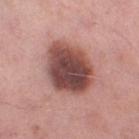{"biopsy_status": "not biopsied; imaged during a skin examination", "lesion_size": {"long_diameter_mm_approx": 6.5}, "lighting": "white-light", "image": {"source": "total-body photography crop", "field_of_view_mm": 15}, "patient": {"sex": "male", "age_approx": 55}, "site": "right lower leg", "automated_metrics": {"area_mm2_approx": 25.0, "eccentricity": 0.65, "cielab_L": 47, "cielab_a": 23, "cielab_b": 22, "vs_skin_contrast_norm": 12.0, "border_irregularity_0_10": 1.5, "color_variation_0_10": 7.0, "peripheral_color_asymmetry": 2.0, "nevus_likeness_0_100": 10, "lesion_detection_confidence_0_100": 100}}